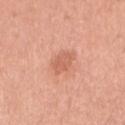No biopsy was performed on this lesion — it was imaged during a full skin examination and was not determined to be concerning. Imaged with white-light lighting. The patient is a female aged around 35. Located on the right upper arm. Cropped from a whole-body photographic skin survey; the tile spans about 15 mm.